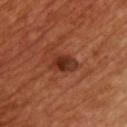TBP lesion metrics = roughly 11 lightness units darker than nearby skin and a normalized border contrast of about 9; a border-irregularity rating of about 3/10, internal color variation of about 6.5 on a 0–10 scale, and radial color variation of about 2
diameter = ≈3.5 mm
image source = total-body-photography crop, ~15 mm field of view
subject = male, in their 50s
anatomic site = the chest
illumination = cross-polarized